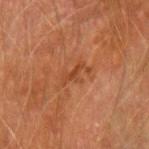workup: total-body-photography surveillance lesion; no biopsy.